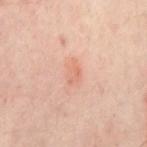biopsy status: no biopsy performed (imaged during a skin exam) | image source: 15 mm crop, total-body photography | location: the mid back | lesion diameter: ≈2.5 mm | patient: aged approximately 55 | lighting: cross-polarized illumination.